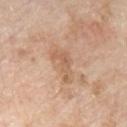Assessment:
No biopsy was performed on this lesion — it was imaged during a full skin examination and was not determined to be concerning.
Image and clinical context:
A 15 mm close-up tile from a total-body photography series done for melanoma screening. The recorded lesion diameter is about 4.5 mm. Captured under white-light illumination. The subject is a male aged 58–62. From the chest. An algorithmic analysis of the crop reported an area of roughly 5.5 mm², a shape eccentricity near 0.9, and a shape-asymmetry score of about 0.55 (0 = symmetric). It also reported a mean CIELAB color near L≈60 a*≈19 b*≈33, a lesion–skin lightness drop of about 8, and a lesion-to-skin contrast of about 6 (normalized; higher = more distinct). The analysis additionally found a border-irregularity rating of about 7/10, a within-lesion color-variation index near 1.5/10, and radial color variation of about 0.5. The analysis additionally found an automated nevus-likeness rating near 0 out of 100 and a detector confidence of about 100 out of 100 that the crop contains a lesion.